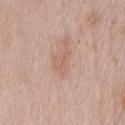Assessment: Captured during whole-body skin photography for melanoma surveillance; the lesion was not biopsied. Acquisition and patient details: Captured under white-light illumination. The lesion's longest dimension is about 3 mm. This image is a 15 mm lesion crop taken from a total-body photograph. A male patient, aged 58–62. The lesion is located on the front of the torso.